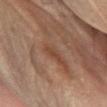Q: Was this lesion biopsied?
A: no biopsy performed (imaged during a skin exam)
Q: What are the patient's age and sex?
A: male, aged approximately 55
Q: What did automated image analysis measure?
A: an area of roughly 2 mm², an eccentricity of roughly 0.95, and two-axis asymmetry of about 0.3; about 6 CIELAB-L* units darker than the surrounding skin; a within-lesion color-variation index near 0/10 and a peripheral color-asymmetry measure near 0
Q: What is the imaging modality?
A: total-body-photography crop, ~15 mm field of view
Q: What is the anatomic site?
A: the right lower leg
Q: Illumination type?
A: cross-polarized
Q: How large is the lesion?
A: about 2.5 mm